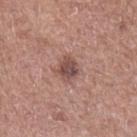biopsy_status: not biopsied; imaged during a skin examination
image:
  source: total-body photography crop
  field_of_view_mm: 15
site: left forearm
lesion_size:
  long_diameter_mm_approx: 2.5
patient:
  sex: male
  age_approx: 65
lighting: white-light